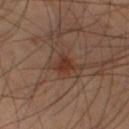Context:
This is a cross-polarized tile. About 3 mm across. The lesion is on the left thigh. A region of skin cropped from a whole-body photographic capture, roughly 15 mm wide. The subject is a male aged 68 to 72.